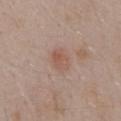Impression:
Captured during whole-body skin photography for melanoma surveillance; the lesion was not biopsied.
Clinical summary:
A close-up tile cropped from a whole-body skin photograph, about 15 mm across. Captured under white-light illumination. The lesion-visualizer software estimated an area of roughly 5.5 mm², a shape eccentricity near 0.7, and a shape-asymmetry score of about 0.2 (0 = symmetric). The analysis additionally found a mean CIELAB color near L≈55 a*≈20 b*≈26, a lesion–skin lightness drop of about 7, and a normalized lesion–skin contrast near 5.5. The lesion is located on the mid back. The patient is a male roughly 55 years of age.